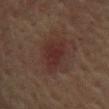acquisition = 15 mm crop, total-body photography | body site = the chest | TBP lesion metrics = a mean CIELAB color near L≈25 a*≈18 b*≈19, about 6 CIELAB-L* units darker than the surrounding skin, and a normalized lesion–skin contrast near 6.5; a border-irregularity rating of about 2.5/10, a within-lesion color-variation index near 2/10, and peripheral color asymmetry of about 0.5; a lesion-detection confidence of about 100/100 | tile lighting = cross-polarized illumination | subject = male, roughly 65 years of age.